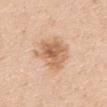This lesion was catalogued during total-body skin photography and was not selected for biopsy.